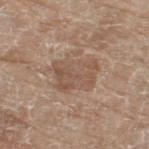workup: imaged on a skin check; not biopsied | patient: female, in their mid- to late 70s | anatomic site: the right thigh | imaging modality: ~15 mm crop, total-body skin-cancer survey.